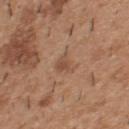Imaged during a routine full-body skin examination; the lesion was not biopsied and no histopathology is available.
A 15 mm close-up extracted from a 3D total-body photography capture.
The lesion is located on the upper back.
A male subject, roughly 40 years of age.